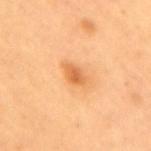<lesion>
  <biopsy_status>not biopsied; imaged during a skin examination</biopsy_status>
  <lesion_size>
    <long_diameter_mm_approx>3.0</long_diameter_mm_approx>
  </lesion_size>
  <lighting>cross-polarized</lighting>
  <site>mid back</site>
  <image>
    <source>total-body photography crop</source>
    <field_of_view_mm>15</field_of_view_mm>
  </image>
  <patient>
    <sex>female</sex>
    <age_approx>70</age_approx>
  </patient>
</lesion>